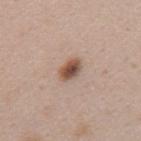Captured during whole-body skin photography for melanoma surveillance; the lesion was not biopsied.
The patient is a female aged around 30.
From the mid back.
A roughly 15 mm field-of-view crop from a total-body skin photograph.